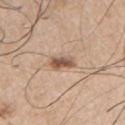– size · ~3.5 mm (longest diameter)
– lighting · white-light
– subject · male, about 75 years old
– anatomic site · the right upper arm
– acquisition · total-body-photography crop, ~15 mm field of view
– TBP lesion metrics · a border-irregularity index near 3.5/10, a within-lesion color-variation index near 5.5/10, and a peripheral color-asymmetry measure near 2; a nevus-likeness score of about 90/100 and a lesion-detection confidence of about 100/100